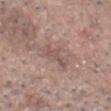Impression:
No biopsy was performed on this lesion — it was imaged during a full skin examination and was not determined to be concerning.
Clinical summary:
A male subject aged around 70. The total-body-photography lesion software estimated a footprint of about 5.5 mm² and an outline eccentricity of about 0.9 (0 = round, 1 = elongated). The lesion is located on the chest. This image is a 15 mm lesion crop taken from a total-body photograph.